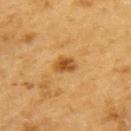biopsy_status: not biopsied; imaged during a skin examination
lighting: cross-polarized
patient:
  sex: male
  age_approx: 85+
lesion_size:
  long_diameter_mm_approx: 2.5
image:
  source: total-body photography crop
  field_of_view_mm: 15
site: upper back
automated_metrics:
  area_mm2_approx: 4.0
  eccentricity: 0.75
  nevus_likeness_0_100: 80
  lesion_detection_confidence_0_100: 100